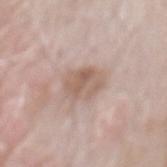Background:
Approximately 3.5 mm at its widest. Imaged with white-light lighting. An algorithmic analysis of the crop reported a lesion area of about 9 mm², a shape eccentricity near 0.55, and two-axis asymmetry of about 0.15. It also reported a mean CIELAB color near L≈59 a*≈16 b*≈25, a lesion–skin lightness drop of about 9, and a normalized border contrast of about 6.5. And it measured a border-irregularity index near 2/10, a within-lesion color-variation index near 4.5/10, and peripheral color asymmetry of about 1.5. A male patient roughly 60 years of age. A 15 mm close-up tile from a total-body photography series done for melanoma screening. On the back.